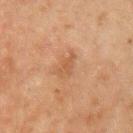biopsy status — imaged on a skin check; not biopsied
diameter — ≈3.5 mm
image source — ~15 mm tile from a whole-body skin photo
TBP lesion metrics — internal color variation of about 0.5 on a 0–10 scale and radial color variation of about 0
illumination — cross-polarized illumination
subject — male, approximately 60 years of age
body site — the left upper arm